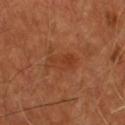No biopsy was performed on this lesion — it was imaged during a full skin examination and was not determined to be concerning. Longest diameter approximately 4 mm. On the chest. Cropped from a total-body skin-imaging series; the visible field is about 15 mm. The subject is a male aged 53–57. Automated image analysis of the tile measured a mean CIELAB color near L≈37 a*≈26 b*≈33 and a lesion-to-skin contrast of about 5 (normalized; higher = more distinct).